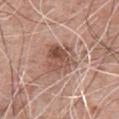{"biopsy_status": "not biopsied; imaged during a skin examination", "lesion_size": {"long_diameter_mm_approx": 4.5}, "site": "chest", "image": {"source": "total-body photography crop", "field_of_view_mm": 15}, "patient": {"sex": "male", "age_approx": 65}}